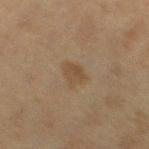| key | value |
|---|---|
| notes | catalogued during a skin exam; not biopsied |
| patient | female, aged around 40 |
| acquisition | ~15 mm tile from a whole-body skin photo |
| location | the right lower leg |
| size | about 3 mm |
| lighting | cross-polarized illumination |